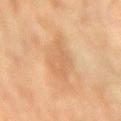follow-up: catalogued during a skin exam; not biopsied
location: the arm
diameter: ~6 mm (longest diameter)
patient: male, about 75 years old
automated metrics: a footprint of about 11 mm², an outline eccentricity of about 0.9 (0 = round, 1 = elongated), and two-axis asymmetry of about 0.35; a mean CIELAB color near L≈54 a*≈18 b*≈33 and a lesion–skin lightness drop of about 6; a classifier nevus-likeness of about 0/100
image: ~15 mm crop, total-body skin-cancer survey
lighting: cross-polarized illumination